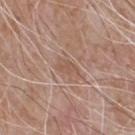* biopsy status: no biopsy performed (imaged during a skin exam)
* illumination: white-light
* subject: male, roughly 65 years of age
* TBP lesion metrics: a lesion color around L≈54 a*≈19 b*≈28 in CIELAB, roughly 6 lightness units darker than nearby skin, and a normalized lesion–skin contrast near 5
* lesion size: ≈2.5 mm
* body site: the chest
* image source: 15 mm crop, total-body photography